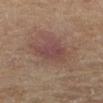{
  "biopsy_status": "not biopsied; imaged during a skin examination",
  "image": {
    "source": "total-body photography crop",
    "field_of_view_mm": 15
  },
  "site": "left lower leg",
  "patient": {
    "sex": "female",
    "age_approx": 55
  }
}